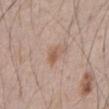| field | value |
|---|---|
| notes | no biopsy performed (imaged during a skin exam) |
| anatomic site | the chest |
| image source | 15 mm crop, total-body photography |
| subject | male, aged approximately 65 |
| lighting | white-light illumination |
| lesion diameter | about 2.5 mm |